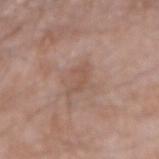A male subject roughly 60 years of age. Cropped from a whole-body photographic skin survey; the tile spans about 15 mm. The tile uses white-light illumination. The lesion is located on the arm. Approximately 3 mm at its widest.